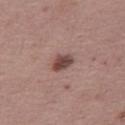No biopsy was performed on this lesion — it was imaged during a full skin examination and was not determined to be concerning.
Measured at roughly 2.5 mm in maximum diameter.
Imaged with white-light lighting.
Automated tile analysis of the lesion measured an eccentricity of roughly 0.6 and a shape-asymmetry score of about 0.3 (0 = symmetric). It also reported internal color variation of about 3 on a 0–10 scale and peripheral color asymmetry of about 1.
A female subject, aged 48–52.
Located on the right thigh.
Cropped from a whole-body photographic skin survey; the tile spans about 15 mm.